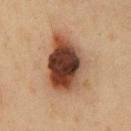workup=catalogued during a skin exam; not biopsied | anatomic site=the front of the torso | acquisition=15 mm crop, total-body photography | subject=male, roughly 50 years of age | size=≈7.5 mm.